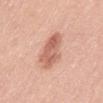<case>
<biopsy_status>not biopsied; imaged during a skin examination</biopsy_status>
<site>abdomen</site>
<image>
  <source>total-body photography crop</source>
  <field_of_view_mm>15</field_of_view_mm>
</image>
<automated_metrics>
  <area_mm2_approx>12.0</area_mm2_approx>
  <eccentricity>0.85</eccentricity>
  <shape_asymmetry>0.2</shape_asymmetry>
  <cielab_L>62</cielab_L>
  <cielab_a>24</cielab_a>
  <cielab_b>30</cielab_b>
  <vs_skin_darker_L>12.0</vs_skin_darker_L>
  <border_irregularity_0_10>3.0</border_irregularity_0_10>
  <color_variation_0_10>4.0</color_variation_0_10>
  <peripheral_color_asymmetry>1.5</peripheral_color_asymmetry>
  <nevus_likeness_0_100>40</nevus_likeness_0_100>
  <lesion_detection_confidence_0_100>100</lesion_detection_confidence_0_100>
</automated_metrics>
<lighting>white-light</lighting>
<patient>
  <sex>female</sex>
  <age_approx>40</age_approx>
</patient>
<lesion_size>
  <long_diameter_mm_approx>5.5</long_diameter_mm_approx>
</lesion_size>
</case>